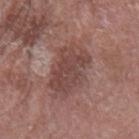Q: Was this lesion biopsied?
A: no biopsy performed (imaged during a skin exam)
Q: Lesion location?
A: the left forearm
Q: Lesion size?
A: about 6.5 mm
Q: Who is the patient?
A: male, in their mid- to late 70s
Q: Illumination type?
A: white-light illumination
Q: What kind of image is this?
A: 15 mm crop, total-body photography